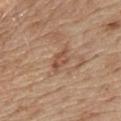biopsy status: catalogued during a skin exam; not biopsied
automated lesion analysis: a lesion area of about 4 mm², an outline eccentricity of about 0.85 (0 = round, 1 = elongated), and a symmetry-axis asymmetry near 0.3; a mean CIELAB color near L≈51 a*≈21 b*≈30, about 9 CIELAB-L* units darker than the surrounding skin, and a normalized lesion–skin contrast near 6.5
patient: male, aged 68 to 72
image source: ~15 mm tile from a whole-body skin photo
site: the chest
illumination: white-light illumination